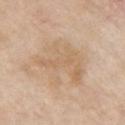On the chest.
A roughly 15 mm field-of-view crop from a total-body skin photograph.
The patient is a female about 70 years old.
The tile uses white-light illumination.
The lesion-visualizer software estimated a mean CIELAB color near L≈64 a*≈15 b*≈33 and a lesion-to-skin contrast of about 4.5 (normalized; higher = more distinct). And it measured a detector confidence of about 100 out of 100 that the crop contains a lesion.
Longest diameter approximately 7 mm.